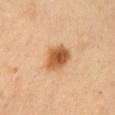workup: catalogued during a skin exam; not biopsied | site: the chest | image source: 15 mm crop, total-body photography | subject: male, in their 50s.